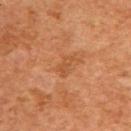Findings:
• biopsy status: imaged on a skin check; not biopsied
• tile lighting: cross-polarized illumination
• patient: male, aged 58–62
• lesion diameter: about 2.5 mm
• automated lesion analysis: a lesion color around L≈51 a*≈26 b*≈39 in CIELAB, about 6 CIELAB-L* units darker than the surrounding skin, and a lesion-to-skin contrast of about 5 (normalized; higher = more distinct); a within-lesion color-variation index near 0.5/10 and radial color variation of about 0
• acquisition: ~15 mm crop, total-body skin-cancer survey
• site: the upper back